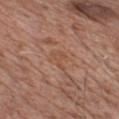follow-up = no biopsy performed (imaged during a skin exam) | automated metrics = a footprint of about 3 mm² and an eccentricity of roughly 0.8; a border-irregularity rating of about 6.5/10 | imaging modality = 15 mm crop, total-body photography | anatomic site = the chest | patient = male, aged 63–67.